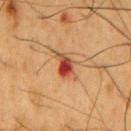notes: no biopsy performed (imaged during a skin exam)
patient: male, in their mid- to late 50s
automated metrics: an average lesion color of about L≈44 a*≈27 b*≈33 (CIELAB), roughly 13 lightness units darker than nearby skin, and a normalized lesion–skin contrast near 10; a border-irregularity rating of about 4/10, a color-variation rating of about 10/10, and peripheral color asymmetry of about 3.5; a classifier nevus-likeness of about 0/100 and a lesion-detection confidence of about 100/100
illumination: cross-polarized
size: ~3.5 mm (longest diameter)
site: the chest
imaging modality: 15 mm crop, total-body photography